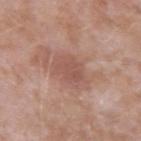acquisition = total-body-photography crop, ~15 mm field of view
automated lesion analysis = a footprint of about 6 mm² and a symmetry-axis asymmetry near 0.25; border irregularity of about 2.5 on a 0–10 scale, a color-variation rating of about 1.5/10, and a peripheral color-asymmetry measure near 0.5; an automated nevus-likeness rating near 0 out of 100 and a detector confidence of about 100 out of 100 that the crop contains a lesion
body site = the right upper arm
subject = male, in their mid-40s
lighting = white-light illumination